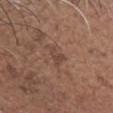Recorded during total-body skin imaging; not selected for excision or biopsy. A region of skin cropped from a whole-body photographic capture, roughly 15 mm wide. A male patient aged around 65. Captured under white-light illumination. The total-body-photography lesion software estimated an outline eccentricity of about 0.7 (0 = round, 1 = elongated) and a shape-asymmetry score of about 0.45 (0 = symmetric). And it measured an average lesion color of about L≈44 a*≈18 b*≈24 (CIELAB), roughly 7 lightness units darker than nearby skin, and a normalized border contrast of about 6. And it measured a border-irregularity rating of about 5/10, a within-lesion color-variation index near 0.5/10, and peripheral color asymmetry of about 0. The analysis additionally found an automated nevus-likeness rating near 0 out of 100. The lesion is located on the head or neck.